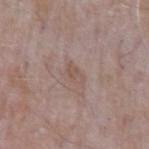biopsy_status: not biopsied; imaged during a skin examination
lesion_size:
  long_diameter_mm_approx: 2.5
patient:
  sex: male
  age_approx: 65
automated_metrics:
  eccentricity: 0.8
  shape_asymmetry: 0.35
  cielab_L: 52
  cielab_a: 16
  cielab_b: 23
  vs_skin_darker_L: 6.0
  vs_skin_contrast_norm: 5.0
  peripheral_color_asymmetry: 0.5
lighting: white-light
site: left upper arm
image:
  source: total-body photography crop
  field_of_view_mm: 15